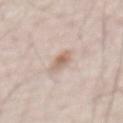Part of a total-body skin-imaging series; this lesion was reviewed on a skin check and was not flagged for biopsy.
Cropped from a total-body skin-imaging series; the visible field is about 15 mm.
Located on the chest.
A male patient, aged around 50.